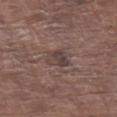workup: catalogued during a skin exam; not biopsied
body site: the left upper arm
diameter: ~2.5 mm (longest diameter)
patient: male, approximately 80 years of age
illumination: white-light
TBP lesion metrics: an outline eccentricity of about 0.5 (0 = round, 1 = elongated) and a symmetry-axis asymmetry near 0.3; a border-irregularity index near 2.5/10, a color-variation rating of about 3.5/10, and radial color variation of about 1.5; an automated nevus-likeness rating near 0 out of 100
acquisition: total-body-photography crop, ~15 mm field of view